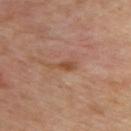| feature | finding |
|---|---|
| follow-up | total-body-photography surveillance lesion; no biopsy |
| image source | ~15 mm tile from a whole-body skin photo |
| subject | female, in their 40s |
| lighting | cross-polarized illumination |
| automated metrics | a shape eccentricity near 0.9 and a symmetry-axis asymmetry near 0.45; a detector confidence of about 100 out of 100 that the crop contains a lesion |
| site | the upper back |